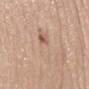{"biopsy_status": "not biopsied; imaged during a skin examination", "site": "mid back", "patient": {"sex": "female", "age_approx": 55}, "lesion_size": {"long_diameter_mm_approx": 7.5}, "image": {"source": "total-body photography crop", "field_of_view_mm": 15}}